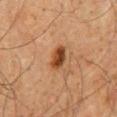Captured during whole-body skin photography for melanoma surveillance; the lesion was not biopsied. The total-body-photography lesion software estimated a lesion color around L≈35 a*≈21 b*≈31 in CIELAB, a lesion–skin lightness drop of about 13, and a lesion-to-skin contrast of about 11 (normalized; higher = more distinct). The software also gave border irregularity of about 1.5 on a 0–10 scale and peripheral color asymmetry of about 1.5. This is a cross-polarized tile. A region of skin cropped from a whole-body photographic capture, roughly 15 mm wide. On the mid back. A male patient in their mid-60s.